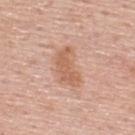notes: total-body-photography surveillance lesion; no biopsy
body site: the upper back
patient: male, approximately 55 years of age
image: ~15 mm tile from a whole-body skin photo
lesion diameter: ~4.5 mm (longest diameter)
lighting: white-light illumination